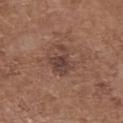Findings:
* subject · female, approximately 75 years of age
* anatomic site · the upper back
* automated lesion analysis · a lesion area of about 7.5 mm² and an eccentricity of roughly 0.65; a lesion color around L≈41 a*≈18 b*≈23 in CIELAB, about 8 CIELAB-L* units darker than the surrounding skin, and a normalized border contrast of about 7.5; border irregularity of about 5.5 on a 0–10 scale, a color-variation rating of about 3.5/10, and a peripheral color-asymmetry measure near 1
* lesion diameter · ~4 mm (longest diameter)
* imaging modality · total-body-photography crop, ~15 mm field of view